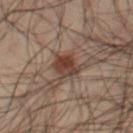No biopsy was performed on this lesion — it was imaged during a full skin examination and was not determined to be concerning.
The lesion is on the right thigh.
Automated image analysis of the tile measured a footprint of about 6.5 mm² and a symmetry-axis asymmetry near 0.2. The software also gave a mean CIELAB color near L≈28 a*≈14 b*≈20 and a normalized lesion–skin contrast near 9.
Cropped from a whole-body photographic skin survey; the tile spans about 15 mm.
A male subject, about 50 years old.
Captured under cross-polarized illumination.